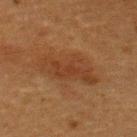Approximately 7 mm at its widest.
This is a cross-polarized tile.
A male patient aged 48–52.
Cropped from a total-body skin-imaging series; the visible field is about 15 mm.
The lesion is located on the upper back.